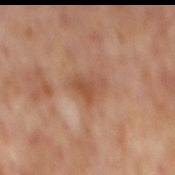Captured during whole-body skin photography for melanoma surveillance; the lesion was not biopsied.
The subject is a male about 65 years old.
Located on the mid back.
Cropped from a total-body skin-imaging series; the visible field is about 15 mm.
An algorithmic analysis of the crop reported a within-lesion color-variation index near 1.5/10 and a peripheral color-asymmetry measure near 0.5. It also reported a classifier nevus-likeness of about 0/100 and a lesion-detection confidence of about 100/100.